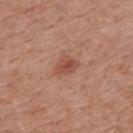biopsy status: catalogued during a skin exam; not biopsied | size: ~2.5 mm (longest diameter) | lighting: white-light illumination | TBP lesion metrics: a footprint of about 4.5 mm² and an eccentricity of roughly 0.7; a detector confidence of about 100 out of 100 that the crop contains a lesion | body site: the upper back | patient: male, about 55 years old | imaging modality: total-body-photography crop, ~15 mm field of view.